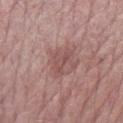Notes:
* notes · total-body-photography surveillance lesion; no biopsy
* tile lighting · white-light
* image · ~15 mm crop, total-body skin-cancer survey
* body site · the left forearm
* automated metrics · a border-irregularity rating of about 4.5/10, a within-lesion color-variation index near 3/10, and radial color variation of about 1; a lesion-detection confidence of about 100/100
* lesion diameter · ~3.5 mm (longest diameter)
* patient · female, in their mid-60s